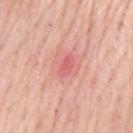{
  "biopsy_status": "not biopsied; imaged during a skin examination",
  "lighting": "white-light",
  "automated_metrics": {
    "cielab_L": 62,
    "cielab_a": 34,
    "cielab_b": 26,
    "vs_skin_darker_L": 9.0
  },
  "image": {
    "source": "total-body photography crop",
    "field_of_view_mm": 15
  },
  "patient": {
    "sex": "male",
    "age_approx": 80
  },
  "lesion_size": {
    "long_diameter_mm_approx": 2.5
  },
  "site": "mid back"
}